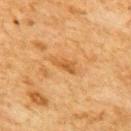No biopsy was performed on this lesion — it was imaged during a full skin examination and was not determined to be concerning.
Imaged with cross-polarized lighting.
The lesion's longest dimension is about 3 mm.
Automated image analysis of the tile measured a border-irregularity index near 4/10 and a color-variation rating of about 1/10. The analysis additionally found a classifier nevus-likeness of about 0/100 and a detector confidence of about 100 out of 100 that the crop contains a lesion.
A roughly 15 mm field-of-view crop from a total-body skin photograph.
A female patient, aged around 60.
From the back.